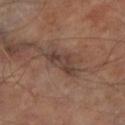Findings:
* biopsy status — catalogued during a skin exam; not biopsied
* subject — male, aged 68–72
* anatomic site — the left lower leg
* acquisition — ~15 mm tile from a whole-body skin photo